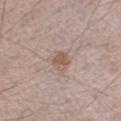Part of a total-body skin-imaging series; this lesion was reviewed on a skin check and was not flagged for biopsy.
Measured at roughly 2.5 mm in maximum diameter.
The patient is a male aged 68 to 72.
On the left upper arm.
A region of skin cropped from a whole-body photographic capture, roughly 15 mm wide.
Imaged with white-light lighting.